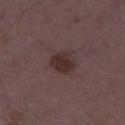Notes:
– tile lighting — white-light illumination
– anatomic site — the leg
– imaging modality — 15 mm crop, total-body photography
– subject — female, aged around 35
– lesion size — ≈3 mm